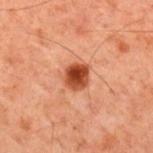Part of a total-body skin-imaging series; this lesion was reviewed on a skin check and was not flagged for biopsy. A male subject, in their 60s. Located on the back. Automated tile analysis of the lesion measured border irregularity of about 1 on a 0–10 scale and a color-variation rating of about 4/10. And it measured a nevus-likeness score of about 100/100 and a detector confidence of about 100 out of 100 that the crop contains a lesion. The tile uses cross-polarized illumination. Cropped from a total-body skin-imaging series; the visible field is about 15 mm.